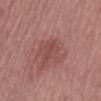<record>
<biopsy_status>not biopsied; imaged during a skin examination</biopsy_status>
<patient>
  <sex>male</sex>
  <age_approx>75</age_approx>
</patient>
<lighting>white-light</lighting>
<automated_metrics>
  <area_mm2_approx>2.0</area_mm2_approx>
  <eccentricity>0.95</eccentricity>
  <shape_asymmetry>0.25</shape_asymmetry>
  <cielab_L>46</cielab_L>
  <cielab_a>26</cielab_a>
  <cielab_b>24</cielab_b>
  <vs_skin_contrast_norm>4.5</vs_skin_contrast_norm>
  <border_irregularity_0_10>3.5</border_irregularity_0_10>
  <color_variation_0_10>0.0</color_variation_0_10>
  <peripheral_color_asymmetry>0.0</peripheral_color_asymmetry>
  <lesion_detection_confidence_0_100>90</lesion_detection_confidence_0_100>
</automated_metrics>
<image>
  <source>total-body photography crop</source>
  <field_of_view_mm>15</field_of_view_mm>
</image>
<site>front of the torso</site>
<lesion_size>
  <long_diameter_mm_approx>3.0</long_diameter_mm_approx>
</lesion_size>
</record>